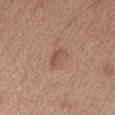workup: catalogued during a skin exam; not biopsied | lesion diameter: about 3 mm | automated lesion analysis: a shape eccentricity near 0.85 and a symmetry-axis asymmetry near 0.35; a border-irregularity index near 3.5/10 and peripheral color asymmetry of about 0.5; an automated nevus-likeness rating near 45 out of 100 and a lesion-detection confidence of about 100/100 | image source: ~15 mm tile from a whole-body skin photo | patient: female, roughly 40 years of age | location: the head or neck | tile lighting: white-light illumination.